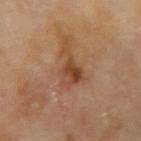Q: Was a biopsy performed?
A: no biopsy performed (imaged during a skin exam)
Q: What is the lesion's diameter?
A: ≈5 mm
Q: Where on the body is the lesion?
A: the right upper arm
Q: What lighting was used for the tile?
A: cross-polarized
Q: What kind of image is this?
A: total-body-photography crop, ~15 mm field of view
Q: Automated lesion metrics?
A: a lesion color around L≈44 a*≈21 b*≈32 in CIELAB, roughly 9 lightness units darker than nearby skin, and a normalized lesion–skin contrast near 7.5
Q: Who is the patient?
A: male, aged 68 to 72